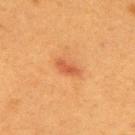biopsy_status: not biopsied; imaged during a skin examination
lesion_size:
  long_diameter_mm_approx: 3.0
patient:
  sex: female
  age_approx: 40
site: back
image:
  source: total-body photography crop
  field_of_view_mm: 15
lighting: cross-polarized
automated_metrics:
  border_irregularity_0_10: 4.0
  color_variation_0_10: 1.0
  peripheral_color_asymmetry: 0.5
  nevus_likeness_0_100: 75
  lesion_detection_confidence_0_100: 100